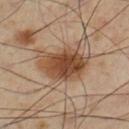Case summary:
• notes: total-body-photography surveillance lesion; no biopsy
• illumination: cross-polarized
• image source: total-body-photography crop, ~15 mm field of view
• patient: male, aged around 55
• anatomic site: the left lower leg
• size: about 6 mm